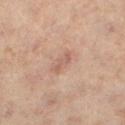Assessment:
Captured during whole-body skin photography for melanoma surveillance; the lesion was not biopsied.
Background:
A female patient aged 53 to 57. This is a cross-polarized tile. A roughly 15 mm field-of-view crop from a total-body skin photograph. Approximately 3 mm at its widest. Located on the left lower leg.